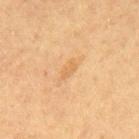Automated tile analysis of the lesion measured border irregularity of about 3.5 on a 0–10 scale and radial color variation of about 0.
Imaged with cross-polarized lighting.
On the mid back.
A roughly 15 mm field-of-view crop from a total-body skin photograph.
Longest diameter approximately 2.5 mm.
A male subject approximately 60 years of age.